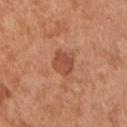Notes:
- biopsy status — total-body-photography surveillance lesion; no biopsy
- image-analysis metrics — a footprint of about 5.5 mm² and a symmetry-axis asymmetry near 0.2; border irregularity of about 2 on a 0–10 scale, a color-variation rating of about 2.5/10, and radial color variation of about 1
- patient — male, in their mid-50s
- tile lighting — white-light illumination
- acquisition — 15 mm crop, total-body photography
- location — the chest
- diameter — ~3 mm (longest diameter)